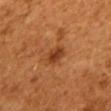Captured during whole-body skin photography for melanoma surveillance; the lesion was not biopsied.
A lesion tile, about 15 mm wide, cut from a 3D total-body photograph.
Longest diameter approximately 2.5 mm.
A male patient, about 45 years old.
The lesion is located on the mid back.
An algorithmic analysis of the crop reported a lesion color around L≈37 a*≈26 b*≈36 in CIELAB and about 10 CIELAB-L* units darker than the surrounding skin. It also reported a nevus-likeness score of about 80/100 and a detector confidence of about 100 out of 100 that the crop contains a lesion.